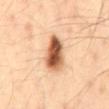Assessment: No biopsy was performed on this lesion — it was imaged during a full skin examination and was not determined to be concerning. Background: A male subject in their 40s. A 15 mm close-up extracted from a 3D total-body photography capture. Located on the mid back. The tile uses cross-polarized illumination.